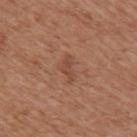This lesion was catalogued during total-body skin photography and was not selected for biopsy. The lesion is on the back. Captured under white-light illumination. This image is a 15 mm lesion crop taken from a total-body photograph. A female patient, in their mid- to late 70s.